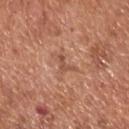Q: Is there a histopathology result?
A: total-body-photography surveillance lesion; no biopsy
Q: Lesion size?
A: ~2.5 mm (longest diameter)
Q: What are the patient's age and sex?
A: male, aged 63–67
Q: Illumination type?
A: white-light illumination
Q: How was this image acquired?
A: 15 mm crop, total-body photography
Q: Lesion location?
A: the upper back
Q: What did automated image analysis measure?
A: a lesion–skin lightness drop of about 8 and a lesion-to-skin contrast of about 6 (normalized; higher = more distinct); a border-irregularity rating of about 7/10, a within-lesion color-variation index near 0/10, and a peripheral color-asymmetry measure near 0; an automated nevus-likeness rating near 0 out of 100